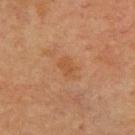Clinical impression:
No biopsy was performed on this lesion — it was imaged during a full skin examination and was not determined to be concerning.
Background:
The patient is a male in their 60s. An algorithmic analysis of the crop reported a mean CIELAB color near L≈43 a*≈19 b*≈31, roughly 5 lightness units darker than nearby skin, and a lesion-to-skin contrast of about 5 (normalized; higher = more distinct). The lesion is on the upper back. Cropped from a whole-body photographic skin survey; the tile spans about 15 mm. About 3 mm across. This is a cross-polarized tile.